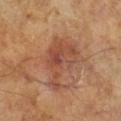The lesion was photographed on a routine skin check and not biopsied; there is no pathology result.
The patient is a male aged 63 to 67.
This image is a 15 mm lesion crop taken from a total-body photograph.
Approximately 6.5 mm at its widest.